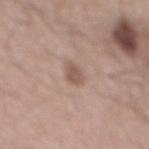Image and clinical context:
From the mid back. A region of skin cropped from a whole-body photographic capture, roughly 15 mm wide. The lesion-visualizer software estimated a border-irregularity rating of about 2/10, a within-lesion color-variation index near 2/10, and a peripheral color-asymmetry measure near 1. It also reported an automated nevus-likeness rating near 25 out of 100 and lesion-presence confidence of about 100/100. The recorded lesion diameter is about 2.5 mm. A male subject aged 53 to 57. The tile uses white-light illumination.